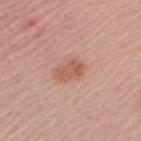biopsy status: total-body-photography surveillance lesion; no biopsy
lesion size: ≈3.5 mm
image: ~15 mm crop, total-body skin-cancer survey
subject: female, aged approximately 60
lighting: white-light illumination
site: the left upper arm